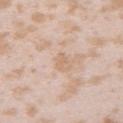Imaged during a routine full-body skin examination; the lesion was not biopsied and no histopathology is available. The tile uses white-light illumination. A region of skin cropped from a whole-body photographic capture, roughly 15 mm wide. The subject is a female about 25 years old. Located on the left upper arm.